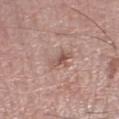Q: Was this lesion biopsied?
A: imaged on a skin check; not biopsied
Q: How was the tile lit?
A: white-light illumination
Q: Automated lesion metrics?
A: a lesion color around L≈57 a*≈18 b*≈24 in CIELAB, roughly 7 lightness units darker than nearby skin, and a normalized border contrast of about 5
Q: What is the lesion's diameter?
A: ≈3.5 mm
Q: What are the patient's age and sex?
A: male, aged 68–72
Q: Lesion location?
A: the right lower leg
Q: How was this image acquired?
A: ~15 mm crop, total-body skin-cancer survey